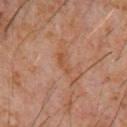This lesion was catalogued during total-body skin photography and was not selected for biopsy. The total-body-photography lesion software estimated an outline eccentricity of about 0.95 (0 = round, 1 = elongated) and a shape-asymmetry score of about 0.4 (0 = symmetric). The analysis additionally found a within-lesion color-variation index near 0/10. This is a cross-polarized tile. The patient is a male aged approximately 60. Approximately 3 mm at its widest. Cropped from a total-body skin-imaging series; the visible field is about 15 mm. From the chest.